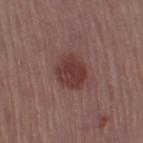{"biopsy_status": "not biopsied; imaged during a skin examination", "lighting": "white-light", "patient": {"sex": "female", "age_approx": 55}, "lesion_size": {"long_diameter_mm_approx": 4.0}, "automated_metrics": {"cielab_L": 38, "cielab_a": 22, "cielab_b": 21, "vs_skin_contrast_norm": 8.0, "border_irregularity_0_10": 2.0, "color_variation_0_10": 3.0, "peripheral_color_asymmetry": 1.0, "nevus_likeness_0_100": 80, "lesion_detection_confidence_0_100": 100}, "image": {"source": "total-body photography crop", "field_of_view_mm": 15}, "site": "right thigh"}